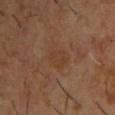Impression:
Part of a total-body skin-imaging series; this lesion was reviewed on a skin check and was not flagged for biopsy.
Context:
Longest diameter approximately 3.5 mm. A male subject in their mid- to late 50s. This image is a 15 mm lesion crop taken from a total-body photograph. An algorithmic analysis of the crop reported a color-variation rating of about 2.5/10 and radial color variation of about 1. The lesion is on the chest.